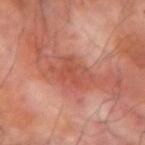The patient is a male aged approximately 70. Located on the left forearm. Longest diameter approximately 5 mm. Cropped from a whole-body photographic skin survey; the tile spans about 15 mm. This is a cross-polarized tile. Automated image analysis of the tile measured a footprint of about 9 mm² and two-axis asymmetry of about 0.35. It also reported a mean CIELAB color near L≈50 a*≈29 b*≈31, about 8 CIELAB-L* units darker than the surrounding skin, and a normalized border contrast of about 6. The software also gave border irregularity of about 5 on a 0–10 scale and a peripheral color-asymmetry measure near 1. The software also gave a lesion-detection confidence of about 100/100.